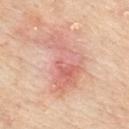Assessment:
Imaged during a routine full-body skin examination; the lesion was not biopsied and no histopathology is available.
Clinical summary:
A roughly 15 mm field-of-view crop from a total-body skin photograph. The lesion is on the upper back. The total-body-photography lesion software estimated a border-irregularity index near 6.5/10, internal color variation of about 7 on a 0–10 scale, and radial color variation of about 2. The lesion's longest dimension is about 7.5 mm. A female patient, aged around 70. This is a white-light tile.